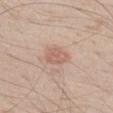workup = imaged on a skin check; not biopsied | lighting = white-light | site = the left thigh | size = about 3 mm | image = total-body-photography crop, ~15 mm field of view | subject = male, aged 63 to 67 | TBP lesion metrics = a lesion area of about 5.5 mm², a shape eccentricity near 0.6, and a symmetry-axis asymmetry near 0.2.